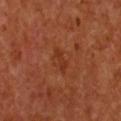Part of a total-body skin-imaging series; this lesion was reviewed on a skin check and was not flagged for biopsy. This is a cross-polarized tile. This image is a 15 mm lesion crop taken from a total-body photograph. The lesion-visualizer software estimated a footprint of about 2.5 mm², a shape eccentricity near 0.95, and a shape-asymmetry score of about 0.25 (0 = symmetric). And it measured a mean CIELAB color near L≈34 a*≈28 b*≈34 and a lesion–skin lightness drop of about 6. The software also gave a classifier nevus-likeness of about 0/100 and lesion-presence confidence of about 100/100. The subject is a female aged 63 to 67. The lesion is located on the chest.